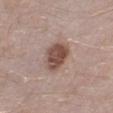| feature | finding |
|---|---|
| biopsy status | imaged on a skin check; not biopsied |
| anatomic site | the left lower leg |
| patient | male, in their 40s |
| size | about 4 mm |
| image source | total-body-photography crop, ~15 mm field of view |